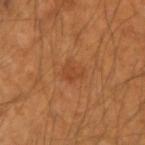patient:
  sex: male
  age_approx: 50
site: left forearm
lesion_size:
  long_diameter_mm_approx: 2.5
image:
  source: total-body photography crop
  field_of_view_mm: 15